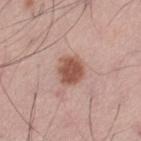{
  "biopsy_status": "not biopsied; imaged during a skin examination",
  "site": "leg",
  "image": {
    "source": "total-body photography crop",
    "field_of_view_mm": 15
  },
  "lighting": "white-light",
  "lesion_size": {
    "long_diameter_mm_approx": 3.0
  },
  "patient": {
    "sex": "male",
    "age_approx": 70
  }
}